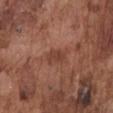Case summary:
• follow-up · no biopsy performed (imaged during a skin exam)
• site · the chest
• TBP lesion metrics · an area of roughly 3.5 mm², an eccentricity of roughly 0.85, and a symmetry-axis asymmetry near 0.35; a lesion color around L≈41 a*≈24 b*≈28 in CIELAB, about 8 CIELAB-L* units darker than the surrounding skin, and a normalized border contrast of about 6.5
• image source · ~15 mm crop, total-body skin-cancer survey
• patient · male, in their mid- to late 70s
• lesion diameter · ≈2.5 mm